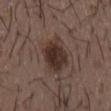No biopsy was performed on this lesion — it was imaged during a full skin examination and was not determined to be concerning.